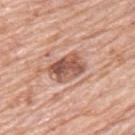{"biopsy_status": "not biopsied; imaged during a skin examination", "lesion_size": {"long_diameter_mm_approx": 4.0}, "site": "left upper arm", "automated_metrics": {"cielab_L": 55, "cielab_a": 23, "cielab_b": 28, "vs_skin_darker_L": 14.0, "vs_skin_contrast_norm": 9.5, "peripheral_color_asymmetry": 2.0, "nevus_likeness_0_100": 55, "lesion_detection_confidence_0_100": 100}, "image": {"source": "total-body photography crop", "field_of_view_mm": 15}, "patient": {"sex": "male", "age_approx": 80}, "lighting": "white-light"}